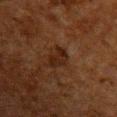Assessment:
Captured during whole-body skin photography for melanoma surveillance; the lesion was not biopsied.
Acquisition and patient details:
About 3.5 mm across. A region of skin cropped from a whole-body photographic capture, roughly 15 mm wide. Automated tile analysis of the lesion measured a footprint of about 5 mm², an eccentricity of roughly 0.85, and a shape-asymmetry score of about 0.3 (0 = symmetric). The software also gave a lesion–skin lightness drop of about 6 and a lesion-to-skin contrast of about 8 (normalized; higher = more distinct). And it measured a nevus-likeness score of about 10/100 and a detector confidence of about 100 out of 100 that the crop contains a lesion. The subject is a male aged around 60. Located on the front of the torso.